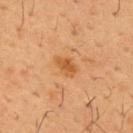Impression: Recorded during total-body skin imaging; not selected for excision or biopsy. Context: The lesion is located on the chest. Measured at roughly 3 mm in maximum diameter. A 15 mm crop from a total-body photograph taken for skin-cancer surveillance. The subject is a male aged around 55.